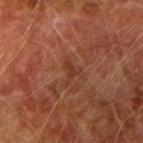- follow-up — imaged on a skin check; not biopsied
- image — 15 mm crop, total-body photography
- lighting — cross-polarized
- patient — male, aged approximately 75
- anatomic site — the arm